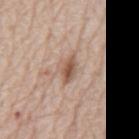| key | value |
|---|---|
| notes | imaged on a skin check; not biopsied |
| anatomic site | the mid back |
| lighting | white-light |
| automated lesion analysis | a border-irregularity index near 2/10 and radial color variation of about 1.5 |
| lesion diameter | ~3.5 mm (longest diameter) |
| patient | male, in their 80s |
| image source | ~15 mm crop, total-body skin-cancer survey |